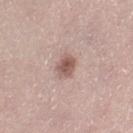Q: Was a biopsy performed?
A: catalogued during a skin exam; not biopsied
Q: What is the anatomic site?
A: the left thigh
Q: How was this image acquired?
A: total-body-photography crop, ~15 mm field of view
Q: Lesion size?
A: about 3 mm
Q: Illumination type?
A: white-light illumination
Q: What are the patient's age and sex?
A: female, roughly 30 years of age